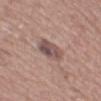Clinical impression:
Part of a total-body skin-imaging series; this lesion was reviewed on a skin check and was not flagged for biopsy.
Clinical summary:
The lesion-visualizer software estimated a border-irregularity index near 2/10, internal color variation of about 6.5 on a 0–10 scale, and radial color variation of about 2.5. And it measured a classifier nevus-likeness of about 5/100 and lesion-presence confidence of about 100/100. The tile uses white-light illumination. A region of skin cropped from a whole-body photographic capture, roughly 15 mm wide. From the mid back. The subject is a male about 75 years old. The lesion's longest dimension is about 3.5 mm.